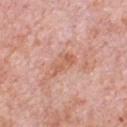A male subject aged 78 to 82. A 15 mm close-up extracted from a 3D total-body photography capture. About 3 mm across. On the chest. The tile uses white-light illumination.